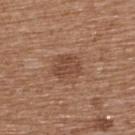| field | value |
|---|---|
| workup | total-body-photography surveillance lesion; no biopsy |
| subject | female, in their mid-70s |
| imaging modality | ~15 mm tile from a whole-body skin photo |
| location | the upper back |
| automated metrics | a footprint of about 7.5 mm² and a symmetry-axis asymmetry near 0.2; a lesion color around L≈46 a*≈21 b*≈29 in CIELAB, a lesion–skin lightness drop of about 8, and a lesion-to-skin contrast of about 6.5 (normalized; higher = more distinct) |
| diameter | ≈3.5 mm |